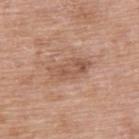workup = imaged on a skin check; not biopsied | size = ≈5 mm | location = the upper back | image = total-body-photography crop, ~15 mm field of view | subject = female, aged approximately 60.